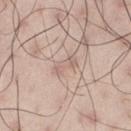The patient is a male about 45 years old.
This is a white-light tile.
Located on the left thigh.
The lesion-visualizer software estimated a classifier nevus-likeness of about 0/100 and lesion-presence confidence of about 80/100.
A roughly 15 mm field-of-view crop from a total-body skin photograph.
Measured at roughly 3 mm in maximum diameter.